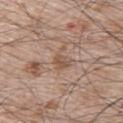Imaged during a routine full-body skin examination; the lesion was not biopsied and no histopathology is available.
From the upper back.
A close-up tile cropped from a whole-body skin photograph, about 15 mm across.
About 2.5 mm across.
A male patient, in their mid-60s.
Captured under white-light illumination.